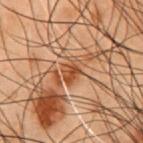Q: Was this lesion biopsied?
A: catalogued during a skin exam; not biopsied
Q: How was this image acquired?
A: ~15 mm tile from a whole-body skin photo
Q: Who is the patient?
A: male, aged approximately 55
Q: What is the anatomic site?
A: the chest
Q: What did automated image analysis measure?
A: an automated nevus-likeness rating near 15 out of 100
Q: What lighting was used for the tile?
A: cross-polarized